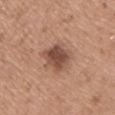Impression: The lesion was tiled from a total-body skin photograph and was not biopsied. Context: The lesion is located on the chest. Longest diameter approximately 4.5 mm. Captured under white-light illumination. An algorithmic analysis of the crop reported border irregularity of about 3 on a 0–10 scale and a within-lesion color-variation index near 4.5/10. Cropped from a whole-body photographic skin survey; the tile spans about 15 mm. The patient is a male in their 50s.